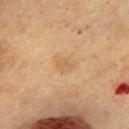Impression:
This lesion was catalogued during total-body skin photography and was not selected for biopsy.
Acquisition and patient details:
On the chest. A female subject aged 58–62. About 3 mm across. This is a cross-polarized tile. The lesion-visualizer software estimated a footprint of about 5 mm² and a symmetry-axis asymmetry near 0.3. And it measured a classifier nevus-likeness of about 5/100 and a detector confidence of about 100 out of 100 that the crop contains a lesion. A 15 mm close-up tile from a total-body photography series done for melanoma screening.